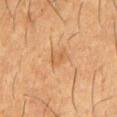Assessment: Recorded during total-body skin imaging; not selected for excision or biopsy. Acquisition and patient details: Longest diameter approximately 2.5 mm. A male subject, in their 60s. The lesion is on the chest. This is a cross-polarized tile. A region of skin cropped from a whole-body photographic capture, roughly 15 mm wide.